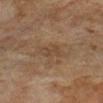Assessment:
Captured during whole-body skin photography for melanoma surveillance; the lesion was not biopsied.
Image and clinical context:
The tile uses cross-polarized illumination. Measured at roughly 2.5 mm in maximum diameter. A lesion tile, about 15 mm wide, cut from a 3D total-body photograph. The patient is a female aged around 70. On the right lower leg.